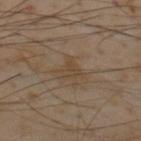This lesion was catalogued during total-body skin photography and was not selected for biopsy. A male subject aged 53 to 57. On the upper back. A roughly 15 mm field-of-view crop from a total-body skin photograph. The lesion-visualizer software estimated a mean CIELAB color near L≈43 a*≈13 b*≈29, a lesion–skin lightness drop of about 6, and a normalized border contrast of about 6. The lesion's longest dimension is about 3 mm. Captured under cross-polarized illumination.